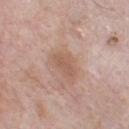This lesion was catalogued during total-body skin photography and was not selected for biopsy. The lesion is on the front of the torso. The patient is a male aged 68 to 72. Captured under white-light illumination. About 3.5 mm across. The total-body-photography lesion software estimated an area of roughly 5 mm² and an outline eccentricity of about 0.85 (0 = round, 1 = elongated). The analysis additionally found a lesion color around L≈57 a*≈19 b*≈29 in CIELAB, roughly 7 lightness units darker than nearby skin, and a normalized border contrast of about 5.5. And it measured border irregularity of about 2.5 on a 0–10 scale, internal color variation of about 1.5 on a 0–10 scale, and peripheral color asymmetry of about 0.5. The analysis additionally found a nevus-likeness score of about 0/100 and a lesion-detection confidence of about 100/100. Cropped from a whole-body photographic skin survey; the tile spans about 15 mm.